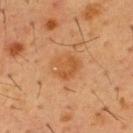No biopsy was performed on this lesion — it was imaged during a full skin examination and was not determined to be concerning. From the chest. A male subject, aged 53 to 57. Cropped from a total-body skin-imaging series; the visible field is about 15 mm.